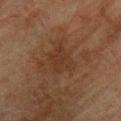  biopsy_status: not biopsied; imaged during a skin examination
  image:
    source: total-body photography crop
    field_of_view_mm: 15
  lighting: cross-polarized
  site: upper back
  patient:
    sex: male
    age_approx: 75
  lesion_size:
    long_diameter_mm_approx: 3.5